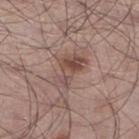This lesion was catalogued during total-body skin photography and was not selected for biopsy. Captured under white-light illumination. Longest diameter approximately 4.5 mm. A 15 mm close-up extracted from a 3D total-body photography capture. A male subject aged 53–57. An algorithmic analysis of the crop reported border irregularity of about 6 on a 0–10 scale and a within-lesion color-variation index near 6/10. The analysis additionally found a nevus-likeness score of about 40/100 and a detector confidence of about 100 out of 100 that the crop contains a lesion. On the leg.